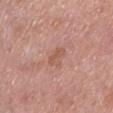Findings:
• biopsy status: catalogued during a skin exam; not biopsied
• imaging modality: ~15 mm crop, total-body skin-cancer survey
• subject: female, aged 48 to 52
• body site: the leg
• tile lighting: white-light illumination